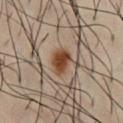body site: the chest; subject: male, aged 38–42; imaging modality: total-body-photography crop, ~15 mm field of view.